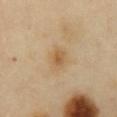No biopsy was performed on this lesion — it was imaged during a full skin examination and was not determined to be concerning. Measured at roughly 2.5 mm in maximum diameter. A female subject aged 58–62. A 15 mm close-up extracted from a 3D total-body photography capture. Imaged with cross-polarized lighting. The lesion is located on the abdomen.